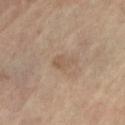Context:
A female patient, about 65 years old. On the left lower leg. The lesion's longest dimension is about 3 mm. Captured under cross-polarized illumination. A 15 mm close-up tile from a total-body photography series done for melanoma screening. The lesion-visualizer software estimated an eccentricity of roughly 0.8 and a symmetry-axis asymmetry near 0.35. It also reported a classifier nevus-likeness of about 0/100 and a detector confidence of about 100 out of 100 that the crop contains a lesion.